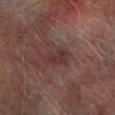Q: Was this lesion biopsied?
A: total-body-photography surveillance lesion; no biopsy
Q: What kind of image is this?
A: ~15 mm crop, total-body skin-cancer survey
Q: What are the patient's age and sex?
A: male, aged 73–77
Q: How large is the lesion?
A: ≈3.5 mm
Q: What did automated image analysis measure?
A: a lesion–skin lightness drop of about 5 and a normalized border contrast of about 6; border irregularity of about 4 on a 0–10 scale and a peripheral color-asymmetry measure near 0.5; a classifier nevus-likeness of about 0/100 and a lesion-detection confidence of about 95/100
Q: Where on the body is the lesion?
A: the right lower leg
Q: What lighting was used for the tile?
A: cross-polarized illumination